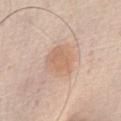Impression:
Part of a total-body skin-imaging series; this lesion was reviewed on a skin check and was not flagged for biopsy.
Background:
Located on the abdomen. Captured under white-light illumination. Automated image analysis of the tile measured an average lesion color of about L≈65 a*≈18 b*≈31 (CIELAB). The analysis additionally found an automated nevus-likeness rating near 55 out of 100 and a lesion-detection confidence of about 100/100. Cropped from a whole-body photographic skin survey; the tile spans about 15 mm. A male subject in their 60s. Approximately 4 mm at its widest.